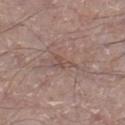workup = total-body-photography surveillance lesion; no biopsy | body site = the leg | patient = male, aged 63 to 67 | automated lesion analysis = internal color variation of about 0 on a 0–10 scale; a detector confidence of about 65 out of 100 that the crop contains a lesion | lesion size = ≈2.5 mm | image = total-body-photography crop, ~15 mm field of view.